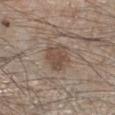The lesion was photographed on a routine skin check and not biopsied; there is no pathology result.
The lesion is located on the right lower leg.
A 15 mm crop from a total-body photograph taken for skin-cancer surveillance.
Captured under white-light illumination.
About 3.5 mm across.
A male patient, about 45 years old.